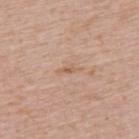No biopsy was performed on this lesion — it was imaged during a full skin examination and was not determined to be concerning.
The lesion is located on the upper back.
Captured under white-light illumination.
A 15 mm crop from a total-body photograph taken for skin-cancer surveillance.
The lesion's longest dimension is about 2.5 mm.
The lesion-visualizer software estimated an area of roughly 2 mm², an outline eccentricity of about 0.95 (0 = round, 1 = elongated), and two-axis asymmetry of about 0.35.
A female patient about 60 years old.